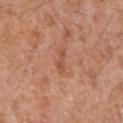biopsy_status: not biopsied; imaged during a skin examination
patient:
  sex: male
  age_approx: 55
lighting: white-light
lesion_size:
  long_diameter_mm_approx: 3.0
site: left upper arm
image:
  source: total-body photography crop
  field_of_view_mm: 15